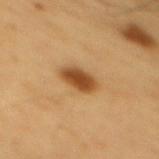{
  "biopsy_status": "not biopsied; imaged during a skin examination"
}